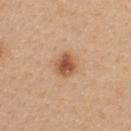Assessment:
Recorded during total-body skin imaging; not selected for excision or biopsy.
Background:
A female patient, aged 23 to 27. A roughly 15 mm field-of-view crop from a total-body skin photograph. The lesion is on the back. Imaged with white-light lighting.